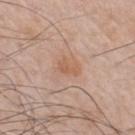- workup: imaged on a skin check; not biopsied
- acquisition: total-body-photography crop, ~15 mm field of view
- body site: the chest
- lesion size: about 2.5 mm
- tile lighting: white-light illumination
- patient: male, aged approximately 50